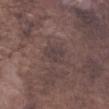follow-up: imaged on a skin check; not biopsied
subject: male, aged around 75
location: the left forearm
automated lesion analysis: a lesion–skin lightness drop of about 5 and a lesion-to-skin contrast of about 5.5 (normalized; higher = more distinct); an automated nevus-likeness rating near 0 out of 100 and a detector confidence of about 60 out of 100 that the crop contains a lesion
diameter: ~3 mm (longest diameter)
image source: total-body-photography crop, ~15 mm field of view